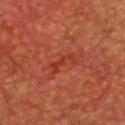Clinical impression:
Captured during whole-body skin photography for melanoma surveillance; the lesion was not biopsied.
Acquisition and patient details:
The lesion-visualizer software estimated a mean CIELAB color near L≈29 a*≈28 b*≈27, a lesion–skin lightness drop of about 5, and a normalized lesion–skin contrast near 5.5. It also reported internal color variation of about 0 on a 0–10 scale and radial color variation of about 0. It also reported an automated nevus-likeness rating near 0 out of 100 and a detector confidence of about 100 out of 100 that the crop contains a lesion. Located on the head or neck. A male patient, aged approximately 60. Longest diameter approximately 3 mm. A 15 mm crop from a total-body photograph taken for skin-cancer surveillance.